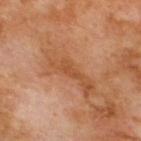Part of a total-body skin-imaging series; this lesion was reviewed on a skin check and was not flagged for biopsy. The lesion-visualizer software estimated a shape eccentricity near 0.9 and a shape-asymmetry score of about 0.45 (0 = symmetric). The analysis additionally found a mean CIELAB color near L≈49 a*≈25 b*≈38 and a lesion-to-skin contrast of about 5.5 (normalized; higher = more distinct). It also reported a border-irregularity rating of about 5/10 and radial color variation of about 0. Imaged with cross-polarized lighting. On the back. Cropped from a total-body skin-imaging series; the visible field is about 15 mm. The subject is a male about 70 years old. The recorded lesion diameter is about 3 mm.